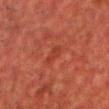Recorded during total-body skin imaging; not selected for excision or biopsy.
From the chest.
A male patient roughly 50 years of age.
A 15 mm crop from a total-body photograph taken for skin-cancer surveillance.
The recorded lesion diameter is about 3 mm.